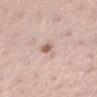Recorded during total-body skin imaging; not selected for excision or biopsy.
Captured under white-light illumination.
The lesion is on the left lower leg.
A female subject about 35 years old.
A close-up tile cropped from a whole-body skin photograph, about 15 mm across.
The lesion's longest dimension is about 4 mm.